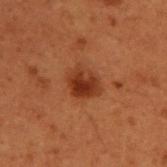Impression:
The lesion was photographed on a routine skin check and not biopsied; there is no pathology result.
Background:
A 15 mm close-up extracted from a 3D total-body photography capture. A male subject in their 50s. This is a cross-polarized tile. The lesion-visualizer software estimated a lesion area of about 8.5 mm², an outline eccentricity of about 0.55 (0 = round, 1 = elongated), and a symmetry-axis asymmetry near 0.2. The analysis additionally found a lesion color around L≈29 a*≈23 b*≈29 in CIELAB and a normalized border contrast of about 8.5. It also reported a border-irregularity index near 2/10, internal color variation of about 5 on a 0–10 scale, and a peripheral color-asymmetry measure near 2. From the upper back. Longest diameter approximately 3.5 mm.